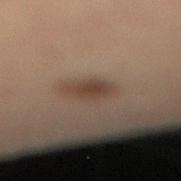This lesion was catalogued during total-body skin photography and was not selected for biopsy.
The total-body-photography lesion software estimated a footprint of about 7 mm² and a shape eccentricity near 0.65. It also reported a lesion color around L≈33 a*≈12 b*≈21 in CIELAB, a lesion–skin lightness drop of about 7, and a normalized lesion–skin contrast near 7.5. It also reported a within-lesion color-variation index near 2/10 and radial color variation of about 0.5.
From the right lower leg.
A male subject in their mid- to late 50s.
A 15 mm close-up tile from a total-body photography series done for melanoma screening.
About 3.5 mm across.